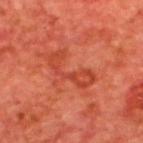Case summary:
* notes — catalogued during a skin exam; not biopsied
* image source — total-body-photography crop, ~15 mm field of view
* diameter — ≈5.5 mm
* illumination — cross-polarized
* TBP lesion metrics — a footprint of about 8.5 mm², an eccentricity of roughly 0.9, and two-axis asymmetry of about 0.6; a mean CIELAB color near L≈42 a*≈35 b*≈35, about 7 CIELAB-L* units darker than the surrounding skin, and a lesion-to-skin contrast of about 5.5 (normalized; higher = more distinct); border irregularity of about 9 on a 0–10 scale, a color-variation rating of about 2/10, and a peripheral color-asymmetry measure near 0.5; a nevus-likeness score of about 0/100 and lesion-presence confidence of about 100/100
* patient — male, aged around 65
* anatomic site — the upper back